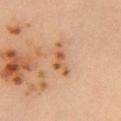{"biopsy_status": "not biopsied; imaged during a skin examination", "automated_metrics": {"cielab_L": 57, "cielab_a": 22, "cielab_b": 37, "vs_skin_darker_L": 10.0, "vs_skin_contrast_norm": 7.5, "border_irregularity_0_10": 7.5, "peripheral_color_asymmetry": 2.5}, "lesion_size": {"long_diameter_mm_approx": 4.5}, "site": "chest", "patient": {"sex": "female", "age_approx": 35}, "image": {"source": "total-body photography crop", "field_of_view_mm": 15}, "lighting": "cross-polarized"}